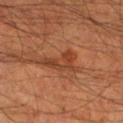notes: total-body-photography surveillance lesion; no biopsy | site: the left lower leg | automated metrics: an outline eccentricity of about 0.9 (0 = round, 1 = elongated) and a symmetry-axis asymmetry near 0.6; a classifier nevus-likeness of about 0/100 and lesion-presence confidence of about 100/100 | acquisition: total-body-photography crop, ~15 mm field of view | patient: male, aged 63 to 67 | lighting: cross-polarized | lesion size: ~4.5 mm (longest diameter).